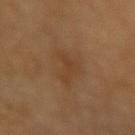Background:
Approximately 4.5 mm at its widest. A female subject aged around 70. Automated tile analysis of the lesion measured a border-irregularity index near 5/10, a within-lesion color-variation index near 2/10, and radial color variation of about 0.5. A 15 mm close-up tile from a total-body photography series done for melanoma screening. Located on the right forearm. Imaged with cross-polarized lighting.